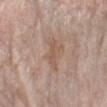Q: Who is the patient?
A: female, about 65 years old
Q: Illumination type?
A: white-light illumination
Q: How was this image acquired?
A: 15 mm crop, total-body photography
Q: What is the anatomic site?
A: the left forearm
Q: How large is the lesion?
A: about 3 mm